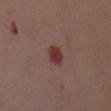No biopsy was performed on this lesion — it was imaged during a full skin examination and was not determined to be concerning. From the chest. Captured under white-light illumination. The subject is a female aged approximately 40. Cropped from a total-body skin-imaging series; the visible field is about 15 mm.